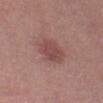Case summary:
• follow-up: imaged on a skin check; not biopsied
• lesion size: ~4.5 mm (longest diameter)
• imaging modality: ~15 mm tile from a whole-body skin photo
• lighting: white-light illumination
• TBP lesion metrics: a lesion color around L≈47 a*≈23 b*≈22 in CIELAB, about 9 CIELAB-L* units darker than the surrounding skin, and a lesion-to-skin contrast of about 6.5 (normalized; higher = more distinct); a within-lesion color-variation index near 2/10 and a peripheral color-asymmetry measure near 0.5; a classifier nevus-likeness of about 80/100 and a detector confidence of about 100 out of 100 that the crop contains a lesion
• subject: female, aged 38–42
• site: the left thigh